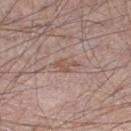Clinical impression:
No biopsy was performed on this lesion — it was imaged during a full skin examination and was not determined to be concerning.